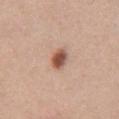Recorded during total-body skin imaging; not selected for excision or biopsy. Measured at roughly 2.5 mm in maximum diameter. Imaged with white-light lighting. A 15 mm close-up tile from a total-body photography series done for melanoma screening. The subject is a female approximately 30 years of age. The lesion is located on the chest. The lesion-visualizer software estimated an area of roughly 4.5 mm², an outline eccentricity of about 0.6 (0 = round, 1 = elongated), and a shape-asymmetry score of about 0.15 (0 = symmetric). And it measured a mean CIELAB color near L≈53 a*≈22 b*≈30 and a normalized border contrast of about 10.5.